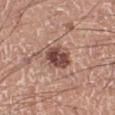Impression:
Imaged during a routine full-body skin examination; the lesion was not biopsied and no histopathology is available.
Image and clinical context:
A male subject aged approximately 60. A region of skin cropped from a whole-body photographic capture, roughly 15 mm wide. The lesion is on the leg.